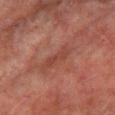* diameter — about 3.5 mm
* patient — male, in their mid-70s
* TBP lesion metrics — an area of roughly 3.5 mm², a shape eccentricity near 0.95, and a shape-asymmetry score of about 0.4 (0 = symmetric); a mean CIELAB color near L≈35 a*≈23 b*≈23 and a lesion–skin lightness drop of about 5; border irregularity of about 5 on a 0–10 scale, a color-variation rating of about 0/10, and a peripheral color-asymmetry measure near 0
* acquisition — total-body-photography crop, ~15 mm field of view
* anatomic site — the leg